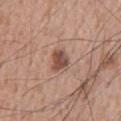Assessment: Recorded during total-body skin imaging; not selected for excision or biopsy. Context: A male patient, approximately 65 years of age. The lesion is on the back. The total-body-photography lesion software estimated about 13 CIELAB-L* units darker than the surrounding skin and a normalized lesion–skin contrast near 9. The analysis additionally found a nevus-likeness score of about 90/100 and lesion-presence confidence of about 100/100. Cropped from a whole-body photographic skin survey; the tile spans about 15 mm. Imaged with white-light lighting. The recorded lesion diameter is about 3 mm.